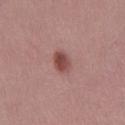A lesion tile, about 15 mm wide, cut from a 3D total-body photograph.
On the leg.
Captured under white-light illumination.
A female subject aged 48 to 52.